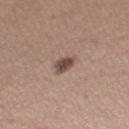| feature | finding |
|---|---|
| anatomic site | the right thigh |
| diameter | about 2.5 mm |
| image source | 15 mm crop, total-body photography |
| image-analysis metrics | a lesion area of about 4 mm² |
| patient | female, aged 28 to 32 |
| illumination | white-light |